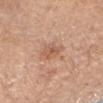A female patient in their mid- to late 60s.
Located on the right forearm.
About 3.5 mm across.
A close-up tile cropped from a whole-body skin photograph, about 15 mm across.
Automated tile analysis of the lesion measured a nevus-likeness score of about 10/100.